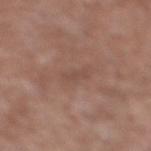Part of a total-body skin-imaging series; this lesion was reviewed on a skin check and was not flagged for biopsy.
A close-up tile cropped from a whole-body skin photograph, about 15 mm across.
This is a white-light tile.
About 3 mm across.
An algorithmic analysis of the crop reported an average lesion color of about L≈47 a*≈19 b*≈24 (CIELAB) and a lesion–skin lightness drop of about 6. It also reported a nevus-likeness score of about 0/100 and a lesion-detection confidence of about 65/100.
The patient is a male aged 58 to 62.
Located on the left lower leg.